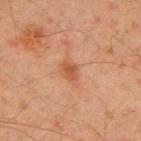Longest diameter approximately 2.5 mm.
Captured under cross-polarized illumination.
On the upper back.
The lesion-visualizer software estimated an average lesion color of about L≈44 a*≈22 b*≈31 (CIELAB), roughly 8 lightness units darker than nearby skin, and a normalized border contrast of about 7. The software also gave a border-irregularity index near 2/10 and radial color variation of about 0.5. The analysis additionally found a nevus-likeness score of about 25/100.
The subject is a male approximately 45 years of age.
A region of skin cropped from a whole-body photographic capture, roughly 15 mm wide.